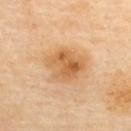follow-up = imaged on a skin check; not biopsied
patient = female, in their 60s
site = the upper back
diameter = about 5 mm
image source = 15 mm crop, total-body photography